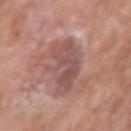Assessment:
This lesion was catalogued during total-body skin photography and was not selected for biopsy.
Background:
A region of skin cropped from a whole-body photographic capture, roughly 15 mm wide. The lesion is located on the arm. A male subject, aged around 60.